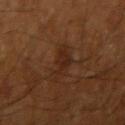<lesion>
<biopsy_status>not biopsied; imaged during a skin examination</biopsy_status>
<lighting>cross-polarized</lighting>
<lesion_size>
  <long_diameter_mm_approx>4.0</long_diameter_mm_approx>
</lesion_size>
<patient>
  <sex>male</sex>
  <age_approx>60</age_approx>
</patient>
<automated_metrics>
  <cielab_L>23</cielab_L>
  <cielab_a>18</cielab_a>
  <cielab_b>25</cielab_b>
  <vs_skin_darker_L>5.0</vs_skin_darker_L>
  <vs_skin_contrast_norm>6.5</vs_skin_contrast_norm>
  <border_irregularity_0_10>5.5</border_irregularity_0_10>
  <color_variation_0_10>2.0</color_variation_0_10>
  <peripheral_color_asymmetry>0.5</peripheral_color_asymmetry>
</automated_metrics>
<site>right upper arm</site>
<image>
  <source>total-body photography crop</source>
  <field_of_view_mm>15</field_of_view_mm>
</image>
</lesion>